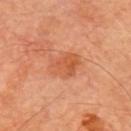Impression:
This lesion was catalogued during total-body skin photography and was not selected for biopsy.
Image and clinical context:
The tile uses cross-polarized illumination. A region of skin cropped from a whole-body photographic capture, roughly 15 mm wide. A male patient, aged approximately 60. Longest diameter approximately 4 mm. On the right upper arm.